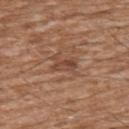| key | value |
|---|---|
| notes | imaged on a skin check; not biopsied |
| imaging modality | total-body-photography crop, ~15 mm field of view |
| site | the front of the torso |
| lighting | white-light illumination |
| patient | male, aged 58 to 62 |
| TBP lesion metrics | a mean CIELAB color near L≈45 a*≈21 b*≈29, roughly 8 lightness units darker than nearby skin, and a lesion-to-skin contrast of about 6 (normalized; higher = more distinct); a detector confidence of about 95 out of 100 that the crop contains a lesion |